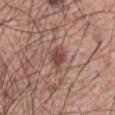Clinical impression:
Captured during whole-body skin photography for melanoma surveillance; the lesion was not biopsied.
Acquisition and patient details:
From the abdomen. A male patient, roughly 60 years of age. About 3.5 mm across. This is a white-light tile. A 15 mm crop from a total-body photograph taken for skin-cancer surveillance. The lesion-visualizer software estimated a lesion area of about 5.5 mm², an eccentricity of roughly 0.75, and a shape-asymmetry score of about 0.25 (0 = symmetric). The analysis additionally found a classifier nevus-likeness of about 75/100 and a lesion-detection confidence of about 100/100.